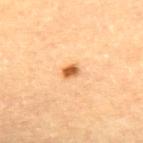<case>
<biopsy_status>not biopsied; imaged during a skin examination</biopsy_status>
<patient>
  <sex>female</sex>
  <age_approx>65</age_approx>
</patient>
<automated_metrics>
  <area_mm2_approx>2.5</area_mm2_approx>
  <eccentricity>0.65</eccentricity>
  <shape_asymmetry>0.2</shape_asymmetry>
  <cielab_L>64</cielab_L>
  <cielab_a>27</cielab_a>
  <cielab_b>47</cielab_b>
  <vs_skin_darker_L>17.0</vs_skin_darker_L>
  <vs_skin_contrast_norm>10.0</vs_skin_contrast_norm>
  <nevus_likeness_0_100>100</nevus_likeness_0_100>
  <lesion_detection_confidence_0_100>100</lesion_detection_confidence_0_100>
</automated_metrics>
<site>back</site>
<lesion_size>
  <long_diameter_mm_approx>2.0</long_diameter_mm_approx>
</lesion_size>
<lighting>cross-polarized</lighting>
<image>
  <source>total-body photography crop</source>
  <field_of_view_mm>15</field_of_view_mm>
</image>
</case>